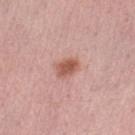Recorded during total-body skin imaging; not selected for excision or biopsy. A 15 mm close-up extracted from a 3D total-body photography capture. Automated image analysis of the tile measured a lesion color around L≈57 a*≈24 b*≈28 in CIELAB and roughly 12 lightness units darker than nearby skin. It also reported internal color variation of about 3 on a 0–10 scale and peripheral color asymmetry of about 1. The software also gave a nevus-likeness score of about 95/100 and a lesion-detection confidence of about 100/100. This is a white-light tile. The lesion is on the left lower leg. Longest diameter approximately 3 mm. A female subject in their mid- to late 50s.